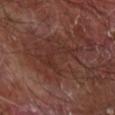Assessment: The lesion was photographed on a routine skin check and not biopsied; there is no pathology result. Image and clinical context: A male subject aged around 65. A roughly 15 mm field-of-view crop from a total-body skin photograph. Captured under cross-polarized illumination. The lesion is located on the arm. Measured at roughly 6 mm in maximum diameter. Automated image analysis of the tile measured a lesion area of about 8 mm², an eccentricity of roughly 0.9, and a shape-asymmetry score of about 0.75 (0 = symmetric). And it measured internal color variation of about 1 on a 0–10 scale.